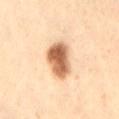Captured during whole-body skin photography for melanoma surveillance; the lesion was not biopsied. The lesion is located on the lower back. Cropped from a whole-body photographic skin survey; the tile spans about 15 mm. A female patient, roughly 40 years of age.